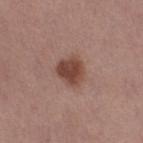workup: no biopsy performed (imaged during a skin exam); patient: female, in their 50s; tile lighting: white-light illumination; anatomic site: the right thigh; acquisition: 15 mm crop, total-body photography.